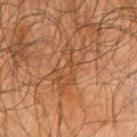The lesion was tiled from a total-body skin photograph and was not biopsied.
From the right upper arm.
Captured under cross-polarized illumination.
Approximately 6 mm at its widest.
A close-up tile cropped from a whole-body skin photograph, about 15 mm across.
An algorithmic analysis of the crop reported a shape eccentricity near 0.9 and a shape-asymmetry score of about 0.45 (0 = symmetric). The analysis additionally found an average lesion color of about L≈47 a*≈22 b*≈35 (CIELAB). It also reported a nevus-likeness score of about 0/100.
A male subject, aged 63 to 67.